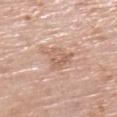Clinical impression:
Part of a total-body skin-imaging series; this lesion was reviewed on a skin check and was not flagged for biopsy.
Acquisition and patient details:
A lesion tile, about 15 mm wide, cut from a 3D total-body photograph. Automated image analysis of the tile measured a lesion–skin lightness drop of about 8 and a normalized border contrast of about 5.5. Located on the left lower leg. A female subject aged 68–72. About 4 mm across. This is a white-light tile.